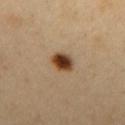| field | value |
|---|---|
| follow-up | no biopsy performed (imaged during a skin exam) |
| diameter | ~3 mm (longest diameter) |
| site | the mid back |
| tile lighting | cross-polarized illumination |
| image | 15 mm crop, total-body photography |
| patient | male, in their 50s |